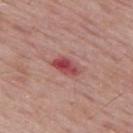Clinical impression:
This lesion was catalogued during total-body skin photography and was not selected for biopsy.
Background:
From the upper back. Longest diameter approximately 3.5 mm. The tile uses white-light illumination. Automated image analysis of the tile measured border irregularity of about 2.5 on a 0–10 scale and peripheral color asymmetry of about 2.5. The analysis additionally found a nevus-likeness score of about 0/100 and a detector confidence of about 100 out of 100 that the crop contains a lesion. A 15 mm close-up tile from a total-body photography series done for melanoma screening. A male patient, aged 63 to 67.